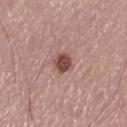No biopsy was performed on this lesion — it was imaged during a full skin examination and was not determined to be concerning. A male subject, aged approximately 55. The recorded lesion diameter is about 2.5 mm. This image is a 15 mm lesion crop taken from a total-body photograph. From the leg.